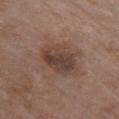The lesion was tiled from a total-body skin photograph and was not biopsied.
Imaged with white-light lighting.
About 5 mm across.
The lesion is located on the chest.
Cropped from a whole-body photographic skin survey; the tile spans about 15 mm.
The patient is a female aged around 85 or older.